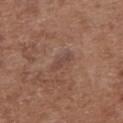Captured during whole-body skin photography for melanoma surveillance; the lesion was not biopsied.
Approximately 2.5 mm at its widest.
This is a white-light tile.
The lesion is located on the upper back.
Cropped from a whole-body photographic skin survey; the tile spans about 15 mm.
A female patient, aged 73 to 77.